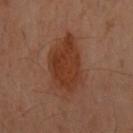Imaged during a routine full-body skin examination; the lesion was not biopsied and no histopathology is available. The recorded lesion diameter is about 6 mm. Cropped from a whole-body photographic skin survey; the tile spans about 15 mm. On the front of the torso. Imaged with cross-polarized lighting. The total-body-photography lesion software estimated an area of roughly 21 mm² and an eccentricity of roughly 0.65. It also reported a mean CIELAB color near L≈31 a*≈20 b*≈27, roughly 8 lightness units darker than nearby skin, and a normalized border contrast of about 8. The analysis additionally found an automated nevus-likeness rating near 95 out of 100 and lesion-presence confidence of about 100/100. A female subject, about 60 years old.